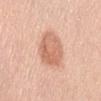Impression:
The lesion was photographed on a routine skin check and not biopsied; there is no pathology result.
Clinical summary:
About 5 mm across. On the abdomen. Imaged with white-light lighting. A region of skin cropped from a whole-body photographic capture, roughly 15 mm wide. A female subject, roughly 60 years of age. An algorithmic analysis of the crop reported a footprint of about 15 mm², a shape eccentricity near 0.7, and two-axis asymmetry of about 0.15. The analysis additionally found a lesion color around L≈66 a*≈23 b*≈32 in CIELAB and a normalized border contrast of about 7. It also reported a border-irregularity rating of about 1.5/10, a within-lesion color-variation index near 4/10, and radial color variation of about 1.5.